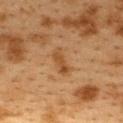Captured during whole-body skin photography for melanoma surveillance; the lesion was not biopsied. A 15 mm close-up tile from a total-body photography series done for melanoma screening. A female subject, aged 38 to 42. The tile uses cross-polarized illumination. Located on the upper back. Automated image analysis of the tile measured an area of roughly 5 mm² and two-axis asymmetry of about 0.2. The software also gave about 7 CIELAB-L* units darker than the surrounding skin and a normalized lesion–skin contrast near 6.5. The software also gave a border-irregularity index near 3/10 and a within-lesion color-variation index near 3/10.